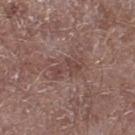Q: What is the lesion's diameter?
A: ~4 mm (longest diameter)
Q: Automated lesion metrics?
A: a mean CIELAB color near L≈44 a*≈19 b*≈21, roughly 7 lightness units darker than nearby skin, and a lesion-to-skin contrast of about 5.5 (normalized; higher = more distinct)
Q: How was the tile lit?
A: white-light illumination
Q: What kind of image is this?
A: 15 mm crop, total-body photography
Q: Lesion location?
A: the right lower leg
Q: Who is the patient?
A: male, aged 68 to 72